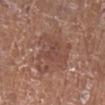Assessment: This lesion was catalogued during total-body skin photography and was not selected for biopsy. Background: A female patient, aged 73–77. Cropped from a whole-body photographic skin survey; the tile spans about 15 mm. Measured at roughly 6 mm in maximum diameter. On the right lower leg.